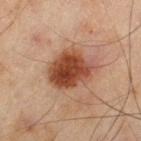Q: Was a biopsy performed?
A: catalogued during a skin exam; not biopsied
Q: Illumination type?
A: cross-polarized illumination
Q: What did automated image analysis measure?
A: a lesion color around L≈36 a*≈21 b*≈27 in CIELAB, roughly 13 lightness units darker than nearby skin, and a lesion-to-skin contrast of about 11 (normalized; higher = more distinct); a classifier nevus-likeness of about 100/100 and lesion-presence confidence of about 100/100
Q: Patient demographics?
A: male, aged approximately 45
Q: Lesion size?
A: ~5.5 mm (longest diameter)
Q: What is the imaging modality?
A: ~15 mm tile from a whole-body skin photo
Q: Where on the body is the lesion?
A: the left thigh